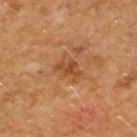Background:
The lesion's longest dimension is about 3 mm. From the upper back. The patient is a male in their 50s. A 15 mm crop from a total-body photograph taken for skin-cancer surveillance.